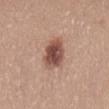image:
  source: total-body photography crop
  field_of_view_mm: 15
lesion_size:
  long_diameter_mm_approx: 4.0
automated_metrics:
  cielab_L: 50
  cielab_a: 22
  cielab_b: 25
  border_irregularity_0_10: 2.0
  color_variation_0_10: 5.0
  nevus_likeness_0_100: 90
  lesion_detection_confidence_0_100: 100
patient:
  sex: female
  age_approx: 35
site: mid back